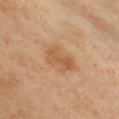| feature | finding |
|---|---|
| workup | no biopsy performed (imaged during a skin exam) |
| subject | female, aged around 40 |
| illumination | cross-polarized |
| automated metrics | an average lesion color of about L≈57 a*≈21 b*≈38 (CIELAB), about 8 CIELAB-L* units darker than the surrounding skin, and a lesion-to-skin contrast of about 6 (normalized; higher = more distinct) |
| lesion diameter | ≈4 mm |
| image source | ~15 mm tile from a whole-body skin photo |
| site | the chest |